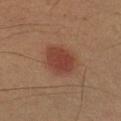{"biopsy_status": "not biopsied; imaged during a skin examination", "site": "left forearm", "lighting": "cross-polarized", "automated_metrics": {"vs_skin_contrast_norm": 7.5}, "patient": {"sex": "male", "age_approx": 40}, "image": {"source": "total-body photography crop", "field_of_view_mm": 15}, "lesion_size": {"long_diameter_mm_approx": 4.5}}